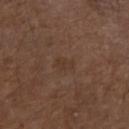biopsy status = no biopsy performed (imaged during a skin exam)
automated metrics = a lesion area of about 3 mm², an eccentricity of roughly 0.8, and two-axis asymmetry of about 0.35; a lesion color around L≈35 a*≈16 b*≈25 in CIELAB, roughly 4 lightness units darker than nearby skin, and a normalized border contrast of about 4.5
image = total-body-photography crop, ~15 mm field of view
anatomic site = the left forearm
lesion diameter = about 2.5 mm
subject = male, aged 73–77
lighting = white-light